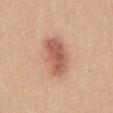Recorded during total-body skin imaging; not selected for excision or biopsy. Measured at roughly 5 mm in maximum diameter. The lesion is on the abdomen. A close-up tile cropped from a whole-body skin photograph, about 15 mm across. The subject is a female aged 18–22.